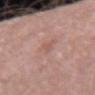patient: female, in their mid- to late 40s; imaging modality: 15 mm crop, total-body photography; site: the right forearm.